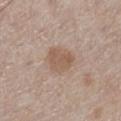  patient:
    sex: male
    age_approx: 70
  lighting: white-light
  site: left lower leg
  automated_metrics:
    border_irregularity_0_10: 2.5
    color_variation_0_10: 2.0
    peripheral_color_asymmetry: 0.5
  image:
    source: total-body photography crop
    field_of_view_mm: 15
  lesion_size:
    long_diameter_mm_approx: 3.5